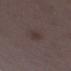Clinical impression: The lesion was tiled from a total-body skin photograph and was not biopsied. Acquisition and patient details: A female patient, about 30 years old. Cropped from a whole-body photographic skin survey; the tile spans about 15 mm. The lesion is on the left lower leg.